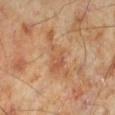This lesion was catalogued during total-body skin photography and was not selected for biopsy. A roughly 15 mm field-of-view crop from a total-body skin photograph. The lesion is located on the left lower leg. Measured at roughly 6 mm in maximum diameter. The patient is a male aged around 70.